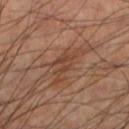This lesion was catalogued during total-body skin photography and was not selected for biopsy. A male subject roughly 60 years of age. The lesion is on the left lower leg. A roughly 15 mm field-of-view crop from a total-body skin photograph.